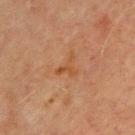{
  "site": "chest",
  "image": {
    "source": "total-body photography crop",
    "field_of_view_mm": 15
  },
  "patient": {
    "sex": "male",
    "age_approx": 70
  }
}